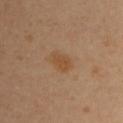Imaged during a routine full-body skin examination; the lesion was not biopsied and no histopathology is available. Imaged with cross-polarized lighting. On the left upper arm. Cropped from a whole-body photographic skin survey; the tile spans about 15 mm. Automated image analysis of the tile measured an eccentricity of roughly 0.8 and a shape-asymmetry score of about 0.25 (0 = symmetric). It also reported a classifier nevus-likeness of about 55/100. The recorded lesion diameter is about 3 mm. A female patient about 40 years old.